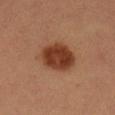  biopsy_status: not biopsied; imaged during a skin examination